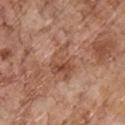{
  "biopsy_status": "not biopsied; imaged during a skin examination",
  "patient": {
    "sex": "male",
    "age_approx": 70
  },
  "lesion_size": {
    "long_diameter_mm_approx": 4.5
  },
  "site": "chest",
  "image": {
    "source": "total-body photography crop",
    "field_of_view_mm": 15
  },
  "automated_metrics": {
    "area_mm2_approx": 10.0,
    "eccentricity": 0.6,
    "shape_asymmetry": 0.45,
    "cielab_L": 50,
    "cielab_a": 21,
    "cielab_b": 31,
    "vs_skin_darker_L": 8.0,
    "vs_skin_contrast_norm": 6.0,
    "nevus_likeness_0_100": 0
  },
  "lighting": "white-light"
}